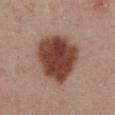Impression: Captured during whole-body skin photography for melanoma surveillance; the lesion was not biopsied. Background: This image is a 15 mm lesion crop taken from a total-body photograph. The lesion is located on the abdomen. A female subject, aged 53 to 57. Captured under white-light illumination.